A 15 mm close-up extracted from a 3D total-body photography capture; the lesion is on the mid back; a male subject, about 50 years old.
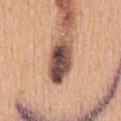<case>
  <diagnosis>
    <histopathology>invasive melanoma, superficial spreading type</histopathology>
    <malignancy>malignant</malignancy>
    <taxonomic_path>Malignant; Malignant melanocytic proliferations (Melanoma); Melanoma Invasive; Melanoma Invasive, Superficial spreading</taxonomic_path>
    <breslow_mm>0.3</breslow_mm>
    <mitotic_index>&lt;1/mm²</mitotic_index>
  </diagnosis>
</case>